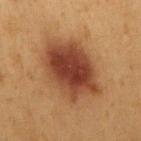The lesion is on the right upper arm. A 15 mm close-up extracted from a 3D total-body photography capture. A male subject in their mid-50s. Longest diameter approximately 7.5 mm. This is a cross-polarized tile.